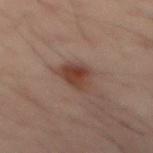The lesion was tiled from a total-body skin photograph and was not biopsied. A region of skin cropped from a whole-body photographic capture, roughly 15 mm wide. The lesion is on the chest. Approximately 3.5 mm at its widest. Captured under cross-polarized illumination. Automated tile analysis of the lesion measured an average lesion color of about L≈31 a*≈16 b*≈21 (CIELAB) and a normalized lesion–skin contrast near 8.5. And it measured lesion-presence confidence of about 100/100. The patient is a male aged approximately 40.